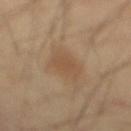lighting: cross-polarized
patient:
  sex: male
  age_approx: 40
image:
  source: total-body photography crop
  field_of_view_mm: 15
site: mid back
lesion_size:
  long_diameter_mm_approx: 4.5
automated_metrics:
  area_mm2_approx: 8.5
  eccentricity: 0.75
  shape_asymmetry: 0.25
  cielab_L: 50
  cielab_a: 16
  cielab_b: 31
  vs_skin_darker_L: 6.0
  vs_skin_contrast_norm: 5.0
  color_variation_0_10: 1.5
  peripheral_color_asymmetry: 0.5